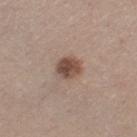| key | value |
|---|---|
| follow-up | imaged on a skin check; not biopsied |
| image | ~15 mm crop, total-body skin-cancer survey |
| tile lighting | white-light |
| body site | the left thigh |
| diameter | ~3 mm (longest diameter) |
| subject | female, approximately 40 years of age |